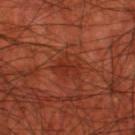| key | value |
|---|---|
| workup | no biopsy performed (imaged during a skin exam) |
| patient | male, aged around 70 |
| site | the left thigh |
| imaging modality | ~15 mm tile from a whole-body skin photo |